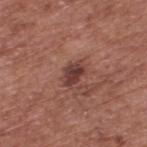Impression:
Imaged during a routine full-body skin examination; the lesion was not biopsied and no histopathology is available.
Clinical summary:
An algorithmic analysis of the crop reported an automated nevus-likeness rating near 25 out of 100 and a lesion-detection confidence of about 100/100. The patient is a male in their mid- to late 70s. The lesion is on the upper back. Measured at roughly 3.5 mm in maximum diameter. This image is a 15 mm lesion crop taken from a total-body photograph. The tile uses white-light illumination.